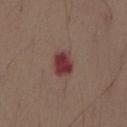Clinical impression: The lesion was tiled from a total-body skin photograph and was not biopsied. Background: Located on the abdomen. A 15 mm crop from a total-body photograph taken for skin-cancer surveillance. A male patient, aged around 75.